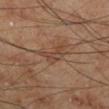{"image": {"source": "total-body photography crop", "field_of_view_mm": 15}, "site": "left lower leg", "patient": {"sex": "male", "age_approx": 70}}